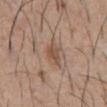Clinical impression:
Imaged during a routine full-body skin examination; the lesion was not biopsied and no histopathology is available.
Clinical summary:
Automated image analysis of the tile measured a lesion color around L≈51 a*≈18 b*≈28 in CIELAB, roughly 8 lightness units darker than nearby skin, and a normalized lesion–skin contrast near 6.5. Captured under white-light illumination. Cropped from a total-body skin-imaging series; the visible field is about 15 mm. The lesion is on the abdomen. About 3 mm across. A male patient in their mid- to late 50s.